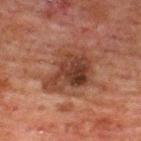workup = total-body-photography surveillance lesion; no biopsy | location = the upper back | subject = male, aged 58 to 62 | imaging modality = total-body-photography crop, ~15 mm field of view.